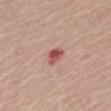Impression: This lesion was catalogued during total-body skin photography and was not selected for biopsy. Acquisition and patient details: Longest diameter approximately 2.5 mm. A close-up tile cropped from a whole-body skin photograph, about 15 mm across. The subject is a female aged 63–67. From the mid back. Captured under white-light illumination.